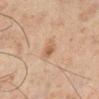<tbp_lesion>
<biopsy_status>not biopsied; imaged during a skin examination</biopsy_status>
<patient>
  <sex>male</sex>
  <age_approx>45</age_approx>
</patient>
<image>
  <source>total-body photography crop</source>
  <field_of_view_mm>15</field_of_view_mm>
</image>
<lesion_size>
  <long_diameter_mm_approx>2.5</long_diameter_mm_approx>
</lesion_size>
<site>right lower leg</site>
<lighting>cross-polarized</lighting>
</tbp_lesion>